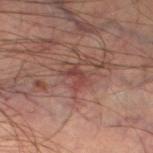Impression: The lesion was photographed on a routine skin check and not biopsied; there is no pathology result. Background: Measured at roughly 2.5 mm in maximum diameter. Captured under cross-polarized illumination. Cropped from a total-body skin-imaging series; the visible field is about 15 mm. A male patient aged approximately 45. Automated image analysis of the tile measured an area of roughly 4 mm² and two-axis asymmetry of about 0.4. And it measured a mean CIELAB color near L≈40 a*≈23 b*≈22, a lesion–skin lightness drop of about 7, and a lesion-to-skin contrast of about 6 (normalized; higher = more distinct). From the left thigh.